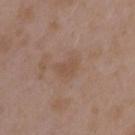The lesion was photographed on a routine skin check and not biopsied; there is no pathology result.
Cropped from a total-body skin-imaging series; the visible field is about 15 mm.
A female subject, in their mid- to late 30s.
The lesion is located on the left upper arm.